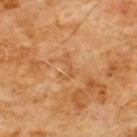Recorded during total-body skin imaging; not selected for excision or biopsy.
The subject is a male aged around 60.
A 15 mm close-up tile from a total-body photography series done for melanoma screening.
Located on the chest.